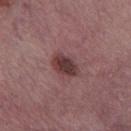Captured during whole-body skin photography for melanoma surveillance; the lesion was not biopsied.
Cropped from a whole-body photographic skin survey; the tile spans about 15 mm.
The lesion is located on the left thigh.
A female subject, aged 53–57.
This is a white-light tile.
The lesion's longest dimension is about 3.5 mm.